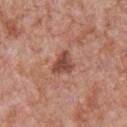<lesion>
<biopsy_status>not biopsied; imaged during a skin examination</biopsy_status>
<patient>
  <sex>male</sex>
  <age_approx>65</age_approx>
</patient>
<image>
  <source>total-body photography crop</source>
  <field_of_view_mm>15</field_of_view_mm>
</image>
<site>chest</site>
</lesion>